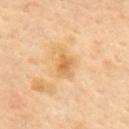Q: Was a biopsy performed?
A: no biopsy performed (imaged during a skin exam)
Q: How was this image acquired?
A: 15 mm crop, total-body photography
Q: Where on the body is the lesion?
A: the upper back
Q: Patient demographics?
A: female, aged approximately 60
Q: What lighting was used for the tile?
A: cross-polarized
Q: What is the lesion's diameter?
A: ≈3.5 mm